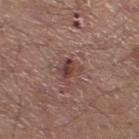Findings:
- notes — no biopsy performed (imaged during a skin exam)
- illumination — white-light illumination
- lesion size — ~3 mm (longest diameter)
- image — 15 mm crop, total-body photography
- body site — the left lower leg
- patient — male, in their mid- to late 50s
- automated metrics — a mean CIELAB color near L≈41 a*≈21 b*≈21 and roughly 10 lightness units darker than nearby skin; a classifier nevus-likeness of about 0/100 and a detector confidence of about 100 out of 100 that the crop contains a lesion